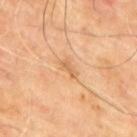Q: Was a biopsy performed?
A: catalogued during a skin exam; not biopsied
Q: Illumination type?
A: cross-polarized
Q: Automated lesion metrics?
A: an area of roughly 2.5 mm² and a symmetry-axis asymmetry near 0.35; a nevus-likeness score of about 0/100
Q: What is the anatomic site?
A: the upper back
Q: Patient demographics?
A: male, in their mid-60s
Q: How large is the lesion?
A: ≈2.5 mm
Q: What is the imaging modality?
A: ~15 mm tile from a whole-body skin photo